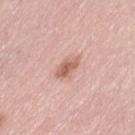Q: Is there a histopathology result?
A: imaged on a skin check; not biopsied
Q: What are the patient's age and sex?
A: female, aged approximately 65
Q: How was the tile lit?
A: white-light illumination
Q: Lesion location?
A: the left thigh
Q: Lesion size?
A: ≈3 mm
Q: Automated lesion metrics?
A: a lesion area of about 4.5 mm², an outline eccentricity of about 0.85 (0 = round, 1 = elongated), and two-axis asymmetry of about 0.25; an average lesion color of about L≈61 a*≈23 b*≈28 (CIELAB), a lesion–skin lightness drop of about 12, and a lesion-to-skin contrast of about 7.5 (normalized; higher = more distinct); a detector confidence of about 100 out of 100 that the crop contains a lesion
Q: What is the imaging modality?
A: ~15 mm tile from a whole-body skin photo